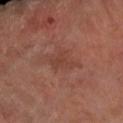{"biopsy_status": "not biopsied; imaged during a skin examination", "automated_metrics": {"vs_skin_darker_L": 5.0, "vs_skin_contrast_norm": 5.0, "border_irregularity_0_10": 4.0, "color_variation_0_10": 1.0, "peripheral_color_asymmetry": 0.5, "nevus_likeness_0_100": 0, "lesion_detection_confidence_0_100": 95}, "site": "left arm", "image": {"source": "total-body photography crop", "field_of_view_mm": 15}, "patient": {"sex": "female", "age_approx": 65}, "lesion_size": {"long_diameter_mm_approx": 3.5}, "lighting": "cross-polarized"}